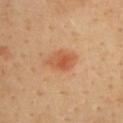Clinical impression: Recorded during total-body skin imaging; not selected for excision or biopsy. Background: The recorded lesion diameter is about 3.5 mm. The patient is a male aged 38 to 42. This image is a 15 mm lesion crop taken from a total-body photograph. Automated tile analysis of the lesion measured a lesion area of about 6.5 mm², an outline eccentricity of about 0.8 (0 = round, 1 = elongated), and a shape-asymmetry score of about 0.3 (0 = symmetric). The lesion is on the upper back.